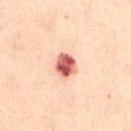The lesion was tiled from a total-body skin photograph and was not biopsied. The lesion is located on the front of the torso. A female subject, aged approximately 55. Cropped from a whole-body photographic skin survey; the tile spans about 15 mm. Captured under cross-polarized illumination.